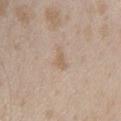Q: Was this lesion biopsied?
A: no biopsy performed (imaged during a skin exam)
Q: What is the imaging modality?
A: ~15 mm tile from a whole-body skin photo
Q: Automated lesion metrics?
A: an area of roughly 3 mm², an eccentricity of roughly 0.8, and a symmetry-axis asymmetry near 0.4; a lesion color around L≈61 a*≈15 b*≈29 in CIELAB, a lesion–skin lightness drop of about 7, and a normalized lesion–skin contrast near 5; a border-irregularity index near 4/10 and a within-lesion color-variation index near 0.5/10
Q: How large is the lesion?
A: ≈2.5 mm
Q: Illumination type?
A: white-light
Q: What is the anatomic site?
A: the upper back
Q: Patient demographics?
A: female, about 25 years old